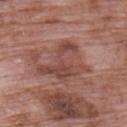Notes:
– follow-up: imaged on a skin check; not biopsied
– image source: ~15 mm tile from a whole-body skin photo
– location: the back
– patient: male, in their 70s
– lesion diameter: about 6 mm